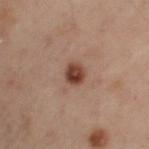{"image": {"source": "total-body photography crop", "field_of_view_mm": 15}, "patient": {"sex": "female", "age_approx": 55}, "site": "left upper arm", "lesion_size": {"long_diameter_mm_approx": 3.0}}